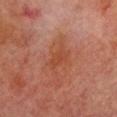The lesion was tiled from a total-body skin photograph and was not biopsied. A female patient aged around 80. The tile uses cross-polarized illumination. Automated tile analysis of the lesion measured a border-irregularity rating of about 3/10 and a within-lesion color-variation index near 1.5/10. And it measured a classifier nevus-likeness of about 0/100 and a detector confidence of about 100 out of 100 that the crop contains a lesion. The lesion is on the chest. A lesion tile, about 15 mm wide, cut from a 3D total-body photograph.